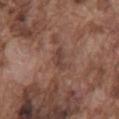patient: male, in their mid-70s; illumination: white-light; diameter: ~3 mm (longest diameter); acquisition: ~15 mm crop, total-body skin-cancer survey; anatomic site: the mid back.